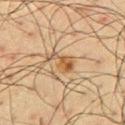Captured during whole-body skin photography for melanoma surveillance; the lesion was not biopsied.